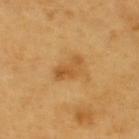notes=no biopsy performed (imaged during a skin exam)
subject=male, aged approximately 60
diameter=≈4 mm
location=the back
image=total-body-photography crop, ~15 mm field of view
lighting=cross-polarized illumination
automated lesion analysis=a lesion area of about 6 mm², an eccentricity of roughly 0.85, and two-axis asymmetry of about 0.4; roughly 8 lightness units darker than nearby skin and a normalized border contrast of about 6; border irregularity of about 4 on a 0–10 scale, a color-variation rating of about 4.5/10, and peripheral color asymmetry of about 2; an automated nevus-likeness rating near 20 out of 100 and lesion-presence confidence of about 100/100